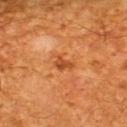Part of a total-body skin-imaging series; this lesion was reviewed on a skin check and was not flagged for biopsy. The patient is a male in their mid- to late 60s. The lesion's longest dimension is about 2.5 mm. The lesion is located on the upper back. This is a cross-polarized tile. A lesion tile, about 15 mm wide, cut from a 3D total-body photograph.